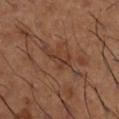biopsy status = no biopsy performed (imaged during a skin exam) | acquisition = total-body-photography crop, ~15 mm field of view | subject = male, aged 63 to 67 | anatomic site = the right lower leg.